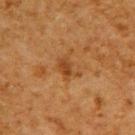follow-up: total-body-photography surveillance lesion; no biopsy
lesion diameter: ≈3 mm
acquisition: ~15 mm crop, total-body skin-cancer survey
site: the upper back
patient: male, roughly 60 years of age
automated lesion analysis: a footprint of about 4.5 mm² and a shape eccentricity near 0.75; an average lesion color of about L≈37 a*≈21 b*≈35 (CIELAB), about 7 CIELAB-L* units darker than the surrounding skin, and a lesion-to-skin contrast of about 6.5 (normalized; higher = more distinct); a classifier nevus-likeness of about 5/100
tile lighting: cross-polarized